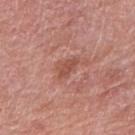Clinical impression:
Imaged during a routine full-body skin examination; the lesion was not biopsied and no histopathology is available.
Clinical summary:
A female subject, approximately 60 years of age. Imaged with white-light lighting. The recorded lesion diameter is about 3 mm. Cropped from a total-body skin-imaging series; the visible field is about 15 mm. The lesion is on the right upper arm. Automated image analysis of the tile measured an area of roughly 4.5 mm² and an outline eccentricity of about 0.8 (0 = round, 1 = elongated). And it measured an average lesion color of about L≈51 a*≈26 b*≈28 (CIELAB) and about 8 CIELAB-L* units darker than the surrounding skin.